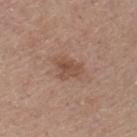* notes: total-body-photography surveillance lesion; no biopsy
* lighting: white-light illumination
* anatomic site: the right lower leg
* diameter: ~4 mm (longest diameter)
* automated metrics: a footprint of about 7 mm², a shape eccentricity near 0.75, and a shape-asymmetry score of about 0.35 (0 = symmetric); a border-irregularity index near 3.5/10, a color-variation rating of about 3/10, and radial color variation of about 1; a nevus-likeness score of about 10/100 and a lesion-detection confidence of about 100/100
* patient: female, about 60 years old
* image: 15 mm crop, total-body photography